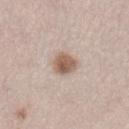notes — imaged on a skin check; not biopsied | subject — female, in their 40s | anatomic site — the right lower leg | tile lighting — white-light | image-analysis metrics — an average lesion color of about L≈59 a*≈16 b*≈26 (CIELAB), roughly 13 lightness units darker than nearby skin, and a normalized lesion–skin contrast near 9; a border-irregularity rating of about 2/10 and peripheral color asymmetry of about 1.5; a classifier nevus-likeness of about 95/100 and a detector confidence of about 100 out of 100 that the crop contains a lesion | imaging modality — total-body-photography crop, ~15 mm field of view | diameter — ≈3 mm.